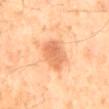Impression:
The lesion was tiled from a total-body skin photograph and was not biopsied.
Context:
The lesion-visualizer software estimated a normalized lesion–skin contrast near 7. And it measured lesion-presence confidence of about 100/100. A male subject, about 55 years old. A 15 mm close-up extracted from a 3D total-body photography capture. The lesion is located on the mid back.